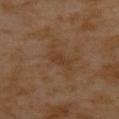Captured during whole-body skin photography for melanoma surveillance; the lesion was not biopsied. The lesion is located on the back. A female subject approximately 55 years of age. Captured under cross-polarized illumination. A roughly 15 mm field-of-view crop from a total-body skin photograph. Approximately 2.5 mm at its widest.